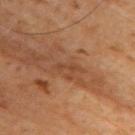anatomic site = the upper back | imaging modality = ~15 mm crop, total-body skin-cancer survey | subject = male, roughly 60 years of age | tile lighting = cross-polarized illumination.